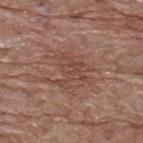Assessment: Recorded during total-body skin imaging; not selected for excision or biopsy. Acquisition and patient details: The lesion is located on the upper back. A male subject, roughly 70 years of age. A 15 mm close-up tile from a total-body photography series done for melanoma screening.